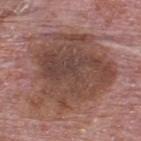Q: Was this lesion biopsied?
A: imaged on a skin check; not biopsied